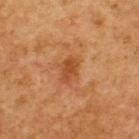Clinical impression: This lesion was catalogued during total-body skin photography and was not selected for biopsy. Background: On the upper back. A male subject, aged 73–77. Imaged with cross-polarized lighting. About 3 mm across. A region of skin cropped from a whole-body photographic capture, roughly 15 mm wide.